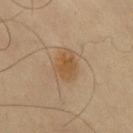No biopsy was performed on this lesion — it was imaged during a full skin examination and was not determined to be concerning. From the upper back. A 15 mm close-up extracted from a 3D total-body photography capture. A male patient aged around 65.